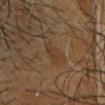Impression: No biopsy was performed on this lesion — it was imaged during a full skin examination and was not determined to be concerning. Clinical summary: A male patient, approximately 65 years of age. On the head or neck. The total-body-photography lesion software estimated a normalized border contrast of about 5. It also reported an automated nevus-likeness rating near 0 out of 100. This image is a 15 mm lesion crop taken from a total-body photograph.